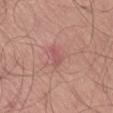<tbp_lesion>
<biopsy_status>not biopsied; imaged during a skin examination</biopsy_status>
<image>
  <source>total-body photography crop</source>
  <field_of_view_mm>15</field_of_view_mm>
</image>
<site>lower back</site>
<patient>
  <sex>male</sex>
  <age_approx>55</age_approx>
</patient>
<lighting>white-light</lighting>
<lesion_size>
  <long_diameter_mm_approx>3.0</long_diameter_mm_approx>
</lesion_size>
</tbp_lesion>